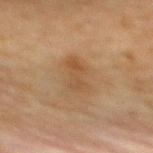Imaged during a routine full-body skin examination; the lesion was not biopsied and no histopathology is available. Cropped from a whole-body photographic skin survey; the tile spans about 15 mm. The lesion's longest dimension is about 4 mm. The patient is a female approximately 70 years of age. The lesion is located on the back.